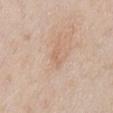Notes:
* subject — male, roughly 65 years of age
* lesion size — ~2 mm (longest diameter)
* image — ~15 mm tile from a whole-body skin photo
* location — the abdomen